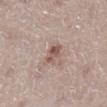follow-up: catalogued during a skin exam; not biopsied
image source: total-body-photography crop, ~15 mm field of view
location: the right lower leg
subject: female, aged 48–52